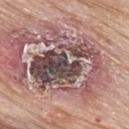{"patient": {"sex": "male", "age_approx": "85+"}, "lesion_size": {"long_diameter_mm_approx": 8.5}, "image": {"source": "total-body photography crop", "field_of_view_mm": 15}, "lighting": "white-light", "site": "back", "diagnosis": {"histopathology": "nodular basal cell carcinoma", "malignancy": "malignant", "taxonomic_path": ["Malignant", "Malignant adnexal epithelial proliferations - Follicular", "Basal cell carcinoma", "Basal cell carcinoma, Nodular"]}}